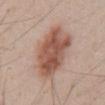Q: What is the imaging modality?
A: ~15 mm tile from a whole-body skin photo
Q: What are the patient's age and sex?
A: male, aged 48 to 52
Q: How was the tile lit?
A: white-light illumination
Q: Lesion location?
A: the abdomen
Q: Automated lesion metrics?
A: an area of roughly 33 mm² and a shape eccentricity near 0.7; a lesion color around L≈56 a*≈20 b*≈27 in CIELAB, a lesion–skin lightness drop of about 12, and a normalized border contrast of about 8
Q: Lesion size?
A: ~8 mm (longest diameter)